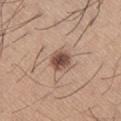  biopsy_status: not biopsied; imaged during a skin examination
  image:
    source: total-body photography crop
    field_of_view_mm: 15
  patient:
    sex: male
    age_approx: 65
  lighting: white-light
  lesion_size:
    long_diameter_mm_approx: 2.5
  site: right thigh
  automated_metrics:
    cielab_L: 50
    cielab_a: 19
    cielab_b: 26
    vs_skin_darker_L: 15.0
    vs_skin_contrast_norm: 10.5